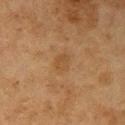Part of a total-body skin-imaging series; this lesion was reviewed on a skin check and was not flagged for biopsy.